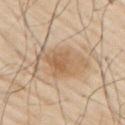The lesion was tiled from a total-body skin photograph and was not biopsied. Automated image analysis of the tile measured a footprint of about 19 mm² and a shape-asymmetry score of about 0.3 (0 = symmetric). And it measured border irregularity of about 5 on a 0–10 scale, a color-variation rating of about 4.5/10, and radial color variation of about 1. It also reported a nevus-likeness score of about 85/100. A male subject about 80 years old. About 7 mm across. On the arm. A 15 mm close-up tile from a total-body photography series done for melanoma screening.